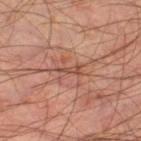Imaged during a routine full-body skin examination; the lesion was not biopsied and no histopathology is available.
A lesion tile, about 15 mm wide, cut from a 3D total-body photograph.
About 3 mm across.
Imaged with cross-polarized lighting.
A male patient, about 45 years old.
Located on the right lower leg.